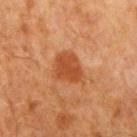Clinical impression:
Recorded during total-body skin imaging; not selected for excision or biopsy.
Acquisition and patient details:
A male subject, approximately 70 years of age. The recorded lesion diameter is about 3.5 mm. From the left upper arm. The total-body-photography lesion software estimated a lesion area of about 9 mm², a shape eccentricity near 0.55, and a shape-asymmetry score of about 0.2 (0 = symmetric). The software also gave an average lesion color of about L≈40 a*≈25 b*≈34 (CIELAB), a lesion–skin lightness drop of about 9, and a lesion-to-skin contrast of about 7.5 (normalized; higher = more distinct). It also reported a border-irregularity rating of about 2/10 and a color-variation rating of about 2/10. The analysis additionally found an automated nevus-likeness rating near 85 out of 100 and a lesion-detection confidence of about 100/100. Cropped from a total-body skin-imaging series; the visible field is about 15 mm. This is a cross-polarized tile.